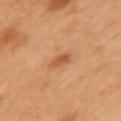Context:
Automated tile analysis of the lesion measured a footprint of about 3.5 mm² and a shape-asymmetry score of about 0.25 (0 = symmetric). And it measured a mean CIELAB color near L≈55 a*≈27 b*≈39, a lesion–skin lightness drop of about 9, and a lesion-to-skin contrast of about 6.5 (normalized; higher = more distinct). Measured at roughly 2.5 mm in maximum diameter. A female patient, roughly 55 years of age. A roughly 15 mm field-of-view crop from a total-body skin photograph. On the left upper arm. The tile uses cross-polarized illumination.